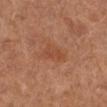Clinical impression:
Captured during whole-body skin photography for melanoma surveillance; the lesion was not biopsied.
Clinical summary:
From the left lower leg. Cropped from a whole-body photographic skin survey; the tile spans about 15 mm. The lesion's longest dimension is about 3.5 mm. Automated tile analysis of the lesion measured a mean CIELAB color near L≈48 a*≈25 b*≈34, roughly 6 lightness units darker than nearby skin, and a normalized lesion–skin contrast near 5. The analysis additionally found a classifier nevus-likeness of about 0/100. A female subject aged 63 to 67.